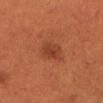Case summary:
– biopsy status · total-body-photography surveillance lesion; no biopsy
– patient · female, approximately 40 years of age
– size · ≈2.5 mm
– image-analysis metrics · an area of roughly 5 mm² and a shape-asymmetry score of about 0.2 (0 = symmetric); a border-irregularity index near 1.5/10 and a color-variation rating of about 2.5/10; a classifier nevus-likeness of about 30/100 and lesion-presence confidence of about 100/100
– acquisition · 15 mm crop, total-body photography
– lighting · cross-polarized
– body site · the head or neck